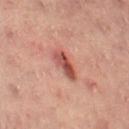{"biopsy_status": "not biopsied; imaged during a skin examination", "patient": {"sex": "female", "age_approx": 55}, "automated_metrics": {"area_mm2_approx": 6.0, "eccentricity": 0.85, "shape_asymmetry": 0.25, "cielab_L": 43, "cielab_a": 25, "cielab_b": 24, "vs_skin_darker_L": 11.0, "peripheral_color_asymmetry": 2.0}, "lesion_size": {"long_diameter_mm_approx": 3.5}, "image": {"source": "total-body photography crop", "field_of_view_mm": 15}, "lighting": "cross-polarized", "site": "left thigh"}